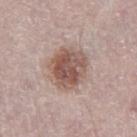notes: catalogued during a skin exam; not biopsied
subject: male, in their mid- to late 70s
location: the leg
acquisition: ~15 mm tile from a whole-body skin photo